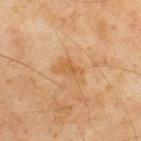Findings:
- notes: catalogued during a skin exam; not biopsied
- tile lighting: cross-polarized
- anatomic site: the back
- acquisition: ~15 mm tile from a whole-body skin photo
- image-analysis metrics: a mean CIELAB color near L≈47 a*≈17 b*≈35 and roughly 6 lightness units darker than nearby skin; a border-irregularity rating of about 4/10
- patient: male, roughly 45 years of age
- lesion diameter: about 3.5 mm